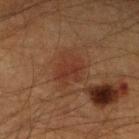subject: male, in their 50s
automated lesion analysis: a border-irregularity index near 3/10, a within-lesion color-variation index near 2.5/10, and a peripheral color-asymmetry measure near 1
tile lighting: cross-polarized illumination
acquisition: ~15 mm crop, total-body skin-cancer survey
body site: the left lower leg